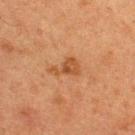follow-up = no biopsy performed (imaged during a skin exam) | anatomic site = the upper back | image-analysis metrics = a footprint of about 5 mm² and an eccentricity of roughly 0.85; a mean CIELAB color near L≈40 a*≈21 b*≈33; a border-irregularity index near 6/10 and internal color variation of about 2 on a 0–10 scale | acquisition = ~15 mm tile from a whole-body skin photo | illumination = cross-polarized | subject = male, aged 58 to 62.